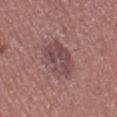Imaged with white-light lighting.
The total-body-photography lesion software estimated an automated nevus-likeness rating near 5 out of 100 and a lesion-detection confidence of about 100/100.
The recorded lesion diameter is about 5 mm.
The lesion is located on the left thigh.
A close-up tile cropped from a whole-body skin photograph, about 15 mm across.
A female patient, aged 38–42.